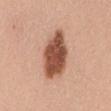Q: Was a biopsy performed?
A: total-body-photography surveillance lesion; no biopsy
Q: Lesion location?
A: the back
Q: How was this image acquired?
A: 15 mm crop, total-body photography
Q: What are the patient's age and sex?
A: female, approximately 50 years of age